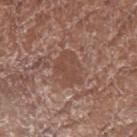The lesion was tiled from a total-body skin photograph and was not biopsied.
Longest diameter approximately 4 mm.
On the right forearm.
Automated image analysis of the tile measured an area of roughly 9.5 mm², an eccentricity of roughly 0.7, and a shape-asymmetry score of about 0.25 (0 = symmetric). It also reported an average lesion color of about L≈46 a*≈20 b*≈26 (CIELAB), roughly 7 lightness units darker than nearby skin, and a lesion-to-skin contrast of about 5.5 (normalized; higher = more distinct). And it measured border irregularity of about 2.5 on a 0–10 scale, internal color variation of about 2 on a 0–10 scale, and radial color variation of about 0.5.
Cropped from a whole-body photographic skin survey; the tile spans about 15 mm.
Captured under white-light illumination.
A female patient aged approximately 75.